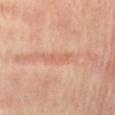Clinical impression:
No biopsy was performed on this lesion — it was imaged during a full skin examination and was not determined to be concerning.
Image and clinical context:
A male subject in their mid-50s. Measured at roughly 3.5 mm in maximum diameter. A region of skin cropped from a whole-body photographic capture, roughly 15 mm wide. From the lower back.